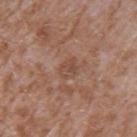biopsy_status: not biopsied; imaged during a skin examination
lesion_size:
  long_diameter_mm_approx: 2.5
lighting: white-light
site: left upper arm
patient:
  sex: male
  age_approx: 45
image:
  source: total-body photography crop
  field_of_view_mm: 15